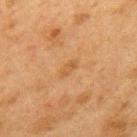notes = no biopsy performed (imaged during a skin exam) | location = the back | lesion size = ≈3 mm | lighting = cross-polarized illumination | acquisition = total-body-photography crop, ~15 mm field of view | image-analysis metrics = a border-irregularity index near 3/10, internal color variation of about 1.5 on a 0–10 scale, and a peripheral color-asymmetry measure near 0; a classifier nevus-likeness of about 0/100 | patient = female, aged 38–42.